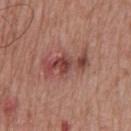workup — total-body-photography surveillance lesion; no biopsy | illumination — white-light illumination | acquisition — ~15 mm crop, total-body skin-cancer survey | body site — the chest | subject — male, in their mid- to late 60s.